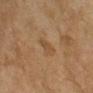biopsy status — no biopsy performed (imaged during a skin exam)
anatomic site — the left upper arm
size — ~2.5 mm (longest diameter)
tile lighting — cross-polarized illumination
subject — female, aged 53–57
image — 15 mm crop, total-body photography